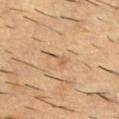Impression:
Captured during whole-body skin photography for melanoma surveillance; the lesion was not biopsied.
Context:
A male subject, about 55 years old. A 15 mm close-up extracted from a 3D total-body photography capture. The lesion is located on the upper back.